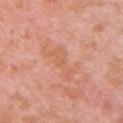Clinical impression:
Imaged during a routine full-body skin examination; the lesion was not biopsied and no histopathology is available.
Clinical summary:
This image is a 15 mm lesion crop taken from a total-body photograph. A female subject about 40 years old. The total-body-photography lesion software estimated a color-variation rating of about 1.5/10 and a peripheral color-asymmetry measure near 0.5. This is a white-light tile. Longest diameter approximately 5.5 mm. On the front of the torso.